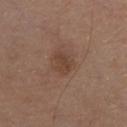{
  "biopsy_status": "not biopsied; imaged during a skin examination",
  "site": "chest",
  "lesion_size": {
    "long_diameter_mm_approx": 2.5
  },
  "lighting": "white-light",
  "patient": {
    "sex": "male",
    "age_approx": 80
  },
  "image": {
    "source": "total-body photography crop",
    "field_of_view_mm": 15
  }
}